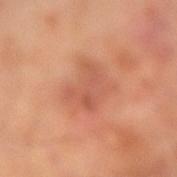| key | value |
|---|---|
| follow-up | imaged on a skin check; not biopsied |
| acquisition | ~15 mm crop, total-body skin-cancer survey |
| site | the arm |
| image-analysis metrics | a mean CIELAB color near L≈55 a*≈27 b*≈33, about 7 CIELAB-L* units darker than the surrounding skin, and a normalized border contrast of about 5; a nevus-likeness score of about 0/100 and a detector confidence of about 100 out of 100 that the crop contains a lesion |
| size | ≈4.5 mm |
| subject | male, in their 70s |
| tile lighting | cross-polarized illumination |